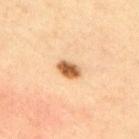Assessment: Imaged during a routine full-body skin examination; the lesion was not biopsied and no histopathology is available. Context: The lesion is located on the upper back. Captured under cross-polarized illumination. A close-up tile cropped from a whole-body skin photograph, about 15 mm across. The lesion's longest dimension is about 3 mm. A male subject about 45 years old.